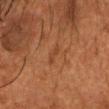{"biopsy_status": "not biopsied; imaged during a skin examination", "patient": {"sex": "male", "age_approx": 60}, "image": {"source": "total-body photography crop", "field_of_view_mm": 15}, "lighting": "cross-polarized", "site": "head or neck", "lesion_size": {"long_diameter_mm_approx": 3.0}}